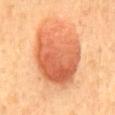Imaged during a routine full-body skin examination; the lesion was not biopsied and no histopathology is available. The subject is a male in their mid-40s. Longest diameter approximately 8 mm. The lesion is on the mid back. A region of skin cropped from a whole-body photographic capture, roughly 15 mm wide. An algorithmic analysis of the crop reported a lesion color around L≈60 a*≈30 b*≈39 in CIELAB, roughly 13 lightness units darker than nearby skin, and a lesion-to-skin contrast of about 8.5 (normalized; higher = more distinct). And it measured border irregularity of about 3 on a 0–10 scale, a color-variation rating of about 7/10, and radial color variation of about 2.5. It also reported an automated nevus-likeness rating near 100 out of 100 and a lesion-detection confidence of about 100/100.